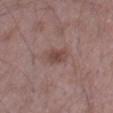imaging modality: 15 mm crop, total-body photography
anatomic site: the right thigh
subject: male, aged approximately 50